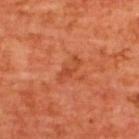biopsy status: no biopsy performed (imaged during a skin exam); image source: 15 mm crop, total-body photography; body site: the upper back; patient: male, aged 63 to 67; tile lighting: cross-polarized.